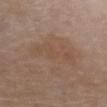Q: Is there a histopathology result?
A: imaged on a skin check; not biopsied
Q: Patient demographics?
A: female, aged 63 to 67
Q: What is the anatomic site?
A: the chest
Q: How was this image acquired?
A: ~15 mm crop, total-body skin-cancer survey